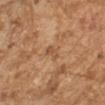{"lesion_size": {"long_diameter_mm_approx": 2.5}, "site": "left forearm", "patient": {"sex": "male", "age_approx": 60}, "image": {"source": "total-body photography crop", "field_of_view_mm": 15}}